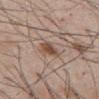Captured during whole-body skin photography for melanoma surveillance; the lesion was not biopsied.